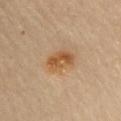Recorded during total-body skin imaging; not selected for excision or biopsy.
A region of skin cropped from a whole-body photographic capture, roughly 15 mm wide.
The lesion is on the chest.
The patient is a male aged 53 to 57.
This is a cross-polarized tile.
Measured at roughly 3.5 mm in maximum diameter.